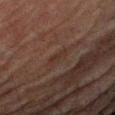<tbp_lesion>
<biopsy_status>not biopsied; imaged during a skin examination</biopsy_status>
<site>right upper arm</site>
<patient>
  <sex>male</sex>
  <age_approx>80</age_approx>
</patient>
<image>
  <source>total-body photography crop</source>
  <field_of_view_mm>15</field_of_view_mm>
</image>
</tbp_lesion>